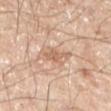Impression: Part of a total-body skin-imaging series; this lesion was reviewed on a skin check and was not flagged for biopsy. Acquisition and patient details: This is a cross-polarized tile. A male subject aged approximately 45. A region of skin cropped from a whole-body photographic capture, roughly 15 mm wide. Automated image analysis of the tile measured a footprint of about 4 mm² and an eccentricity of roughly 0.85. The analysis additionally found a border-irregularity rating of about 4/10, a color-variation rating of about 2/10, and a peripheral color-asymmetry measure near 0.5. The recorded lesion diameter is about 3.5 mm. From the left lower leg.